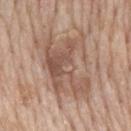| field | value |
|---|---|
| biopsy status | imaged on a skin check; not biopsied |
| automated metrics | a nevus-likeness score of about 0/100 |
| subject | male, aged around 60 |
| acquisition | 15 mm crop, total-body photography |
| tile lighting | white-light illumination |
| lesion size | ≈7.5 mm |
| location | the mid back |